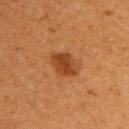| field | value |
|---|---|
| biopsy status | catalogued during a skin exam; not biopsied |
| image | total-body-photography crop, ~15 mm field of view |
| body site | the upper back |
| lighting | cross-polarized illumination |
| patient | female, about 40 years old |
| automated lesion analysis | an area of roughly 8 mm² and a symmetry-axis asymmetry near 0.2; a mean CIELAB color near L≈36 a*≈24 b*≈34 and about 9 CIELAB-L* units darker than the surrounding skin; a border-irregularity index near 2/10, internal color variation of about 4 on a 0–10 scale, and a peripheral color-asymmetry measure near 1 |